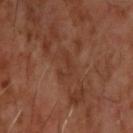biopsy status: imaged on a skin check; not biopsied
illumination: cross-polarized
automated metrics: a lesion area of about 3.5 mm², an eccentricity of roughly 0.85, and a symmetry-axis asymmetry near 0.6; internal color variation of about 0 on a 0–10 scale and a peripheral color-asymmetry measure near 0
imaging modality: 15 mm crop, total-body photography
subject: male, aged around 60
site: the upper back
size: ≈3 mm